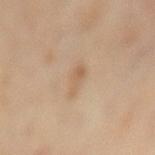follow-up = imaged on a skin check; not biopsied
subject = male, aged 63–67
body site = the lower back
illumination = cross-polarized
acquisition = total-body-photography crop, ~15 mm field of view
automated lesion analysis = an eccentricity of roughly 0.85 and a symmetry-axis asymmetry near 0.55